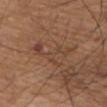Imaged during a routine full-body skin examination; the lesion was not biopsied and no histopathology is available.
A male subject, aged 73–77.
An algorithmic analysis of the crop reported a footprint of about 10 mm² and a shape eccentricity near 0.9. And it measured an average lesion color of about L≈43 a*≈19 b*≈28 (CIELAB), a lesion–skin lightness drop of about 6, and a lesion-to-skin contrast of about 5.5 (normalized; higher = more distinct). The analysis additionally found a nevus-likeness score of about 0/100 and a detector confidence of about 55 out of 100 that the crop contains a lesion.
The tile uses white-light illumination.
Approximately 6.5 mm at its widest.
A roughly 15 mm field-of-view crop from a total-body skin photograph.
From the upper back.